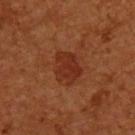Assessment:
Part of a total-body skin-imaging series; this lesion was reviewed on a skin check and was not flagged for biopsy.
Acquisition and patient details:
About 4 mm across. The lesion is located on the upper back. The lesion-visualizer software estimated an average lesion color of about L≈29 a*≈26 b*≈30 (CIELAB), roughly 7 lightness units darker than nearby skin, and a normalized lesion–skin contrast near 7. A roughly 15 mm field-of-view crop from a total-body skin photograph. A male patient, aged approximately 55. This is a cross-polarized tile.